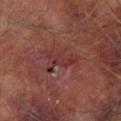Recorded during total-body skin imaging; not selected for excision or biopsy. A male patient, aged 73–77. About 4.5 mm across. On the left lower leg. A 15 mm close-up extracted from a 3D total-body photography capture.